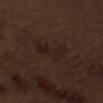Imaged during a routine full-body skin examination; the lesion was not biopsied and no histopathology is available. Imaged with white-light lighting. Longest diameter approximately 5 mm. The subject is a male aged 68–72. The lesion is located on the left upper arm. A region of skin cropped from a whole-body photographic capture, roughly 15 mm wide.